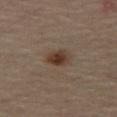Assessment: No biopsy was performed on this lesion — it was imaged during a full skin examination and was not determined to be concerning. Acquisition and patient details: Imaged with cross-polarized lighting. About 3 mm across. The patient is a male in their mid-60s. A close-up tile cropped from a whole-body skin photograph, about 15 mm across. Located on the right thigh.